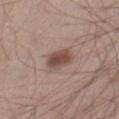The lesion was photographed on a routine skin check and not biopsied; there is no pathology result. From the right thigh. Imaged with white-light lighting. The lesion's longest dimension is about 3.5 mm. A 15 mm close-up extracted from a 3D total-body photography capture. A male subject aged around 60.